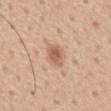Captured under white-light illumination. The lesion is on the mid back. A roughly 15 mm field-of-view crop from a total-body skin photograph. A male subject approximately 55 years of age. The recorded lesion diameter is about 2.5 mm.